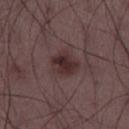Case summary:
– notes · imaged on a skin check; not biopsied
– acquisition · total-body-photography crop, ~15 mm field of view
– site · the left thigh
– diameter · about 3 mm
– image-analysis metrics · an eccentricity of roughly 0.5 and two-axis asymmetry of about 0.2; a nevus-likeness score of about 65/100 and lesion-presence confidence of about 100/100
– tile lighting · white-light
– patient · male, roughly 50 years of age